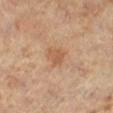Recorded during total-body skin imaging; not selected for excision or biopsy.
The subject is a female aged 63–67.
The lesion is located on the left leg.
A close-up tile cropped from a whole-body skin photograph, about 15 mm across.
This is a cross-polarized tile.
Automated image analysis of the tile measured a classifier nevus-likeness of about 35/100 and a detector confidence of about 100 out of 100 that the crop contains a lesion.
The lesion's longest dimension is about 3 mm.